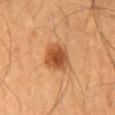The lesion was photographed on a routine skin check and not biopsied; there is no pathology result. A roughly 15 mm field-of-view crop from a total-body skin photograph. A male patient aged 63 to 67. The tile uses cross-polarized illumination. The lesion is located on the mid back.